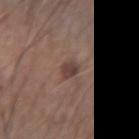follow-up: total-body-photography surveillance lesion; no biopsy | image: 15 mm crop, total-body photography | lighting: white-light | location: the left forearm | patient: male, aged 73 to 77 | lesion diameter: ~2.5 mm (longest diameter).